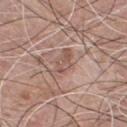Q: Was this lesion biopsied?
A: catalogued during a skin exam; not biopsied
Q: Where on the body is the lesion?
A: the chest
Q: Illumination type?
A: white-light illumination
Q: Automated lesion metrics?
A: an area of roughly 6.5 mm² and a symmetry-axis asymmetry near 0.35; a mean CIELAB color near L≈54 a*≈19 b*≈24 and about 8 CIELAB-L* units darker than the surrounding skin
Q: What kind of image is this?
A: ~15 mm crop, total-body skin-cancer survey
Q: Who is the patient?
A: male, in their mid- to late 70s